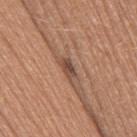Recorded during total-body skin imaging; not selected for excision or biopsy. Measured at roughly 3 mm in maximum diameter. This is a white-light tile. The lesion is located on the leg. The subject is a female approximately 35 years of age. A close-up tile cropped from a whole-body skin photograph, about 15 mm across. An algorithmic analysis of the crop reported an eccentricity of roughly 0.85 and a symmetry-axis asymmetry near 0.3. The analysis additionally found an average lesion color of about L≈47 a*≈20 b*≈27 (CIELAB) and a normalized border contrast of about 8.5.